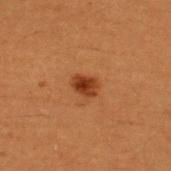Q: Was a biopsy performed?
A: total-body-photography surveillance lesion; no biopsy
Q: How large is the lesion?
A: about 2.5 mm
Q: What did automated image analysis measure?
A: a lesion color around L≈31 a*≈23 b*≈32 in CIELAB, roughly 11 lightness units darker than nearby skin, and a lesion-to-skin contrast of about 10 (normalized; higher = more distinct); border irregularity of about 2 on a 0–10 scale, a color-variation rating of about 4/10, and radial color variation of about 1.5
Q: Patient demographics?
A: male, in their 50s
Q: How was the tile lit?
A: cross-polarized
Q: Lesion location?
A: the upper back
Q: What kind of image is this?
A: 15 mm crop, total-body photography